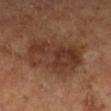| feature | finding |
|---|---|
| workup | no biopsy performed (imaged during a skin exam) |
| automated metrics | a footprint of about 28 mm² and two-axis asymmetry of about 0.25; a lesion-detection confidence of about 100/100 |
| site | the left lower leg |
| subject | male, aged 63 to 67 |
| acquisition | total-body-photography crop, ~15 mm field of view |
| lighting | cross-polarized |
| lesion diameter | about 7 mm |